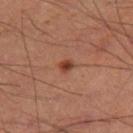Assessment:
The lesion was photographed on a routine skin check and not biopsied; there is no pathology result.
Context:
A 15 mm close-up tile from a total-body photography series done for melanoma screening. The tile uses cross-polarized illumination. The lesion is on the right thigh. A male patient, aged 58–62.